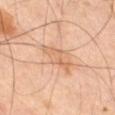| key | value |
|---|---|
| workup | no biopsy performed (imaged during a skin exam) |
| site | the right thigh |
| lighting | cross-polarized |
| patient | male, aged approximately 65 |
| image | 15 mm crop, total-body photography |
| lesion diameter | ~4 mm (longest diameter) |